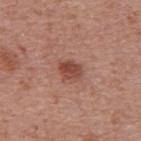biopsy status: catalogued during a skin exam; not biopsied
tile lighting: white-light illumination
subject: male, aged approximately 70
lesion size: ~2.5 mm (longest diameter)
location: the mid back
image: ~15 mm tile from a whole-body skin photo
automated metrics: an outline eccentricity of about 0.65 (0 = round, 1 = elongated) and a symmetry-axis asymmetry near 0.25; an average lesion color of about L≈46 a*≈25 b*≈28 (CIELAB), about 11 CIELAB-L* units darker than the surrounding skin, and a normalized lesion–skin contrast near 8; a border-irregularity rating of about 2.5/10, internal color variation of about 3.5 on a 0–10 scale, and a peripheral color-asymmetry measure near 1.5